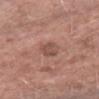Part of a total-body skin-imaging series; this lesion was reviewed on a skin check and was not flagged for biopsy. Measured at roughly 3 mm in maximum diameter. The lesion is located on the arm. This is a white-light tile. An algorithmic analysis of the crop reported a lesion area of about 4.5 mm², a shape eccentricity near 0.75, and two-axis asymmetry of about 0.2. The software also gave roughly 9 lightness units darker than nearby skin and a normalized border contrast of about 6. The analysis additionally found a classifier nevus-likeness of about 0/100 and a lesion-detection confidence of about 100/100. The subject is a female about 65 years old. A 15 mm crop from a total-body photograph taken for skin-cancer surveillance.